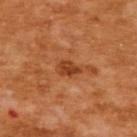workup: total-body-photography surveillance lesion; no biopsy | image-analysis metrics: a footprint of about 4 mm², an eccentricity of roughly 0.75, and a shape-asymmetry score of about 0.3 (0 = symmetric); a mean CIELAB color near L≈43 a*≈30 b*≈41, about 11 CIELAB-L* units darker than the surrounding skin, and a lesion-to-skin contrast of about 8.5 (normalized; higher = more distinct); a peripheral color-asymmetry measure near 1; an automated nevus-likeness rating near 45 out of 100 and lesion-presence confidence of about 100/100 | patient: female, approximately 55 years of age | location: the upper back | imaging modality: ~15 mm crop, total-body skin-cancer survey.